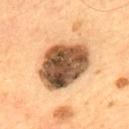{"biopsy_status": "not biopsied; imaged during a skin examination", "lesion_size": {"long_diameter_mm_approx": 7.5}, "image": {"source": "total-body photography crop", "field_of_view_mm": 15}, "site": "upper back", "lighting": "cross-polarized", "patient": {"sex": "male", "age_approx": 55}}